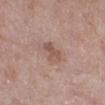  biopsy_status: not biopsied; imaged during a skin examination
  patient:
    sex: female
    age_approx: 70
  lesion_size:
    long_diameter_mm_approx: 3.0
  site: left lower leg
  lighting: white-light
  image:
    source: total-body photography crop
    field_of_view_mm: 15